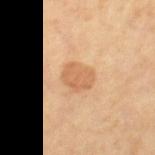Assessment:
Captured during whole-body skin photography for melanoma surveillance; the lesion was not biopsied.
Image and clinical context:
The tile uses cross-polarized illumination. The recorded lesion diameter is about 3.5 mm. The lesion is on the right thigh. A female patient in their mid- to late 60s. A 15 mm close-up extracted from a 3D total-body photography capture.